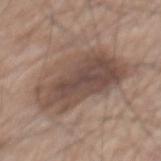The lesion was photographed on a routine skin check and not biopsied; there is no pathology result. Located on the mid back. Measured at roughly 9.5 mm in maximum diameter. A region of skin cropped from a whole-body photographic capture, roughly 15 mm wide. A male patient, in their mid- to late 70s.